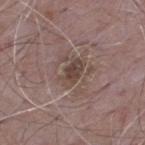Recorded during total-body skin imaging; not selected for excision or biopsy. About 3.5 mm across. This image is a 15 mm lesion crop taken from a total-body photograph. Located on the back. The total-body-photography lesion software estimated a lesion color around L≈43 a*≈15 b*≈21 in CIELAB, a lesion–skin lightness drop of about 9, and a lesion-to-skin contrast of about 7 (normalized; higher = more distinct). It also reported a color-variation rating of about 3/10. A male subject, approximately 65 years of age. Imaged with white-light lighting.